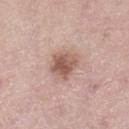The lesion was photographed on a routine skin check and not biopsied; there is no pathology result.
The lesion is located on the left lower leg.
A region of skin cropped from a whole-body photographic capture, roughly 15 mm wide.
A female subject, aged 53 to 57.